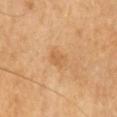| key | value |
|---|---|
| image-analysis metrics | a lesion area of about 4.5 mm², an outline eccentricity of about 0.6 (0 = round, 1 = elongated), and a symmetry-axis asymmetry near 0.2; an average lesion color of about L≈61 a*≈20 b*≈41 (CIELAB), roughly 7 lightness units darker than nearby skin, and a normalized lesion–skin contrast near 5 |
| patient | female, aged 63–67 |
| illumination | cross-polarized |
| anatomic site | the head or neck |
| lesion diameter | about 2.5 mm |
| imaging modality | 15 mm crop, total-body photography |